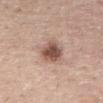Impression: The lesion was tiled from a total-body skin photograph and was not biopsied. Background: A male patient aged 53–57. On the back. Imaged with white-light lighting. This image is a 15 mm lesion crop taken from a total-body photograph.